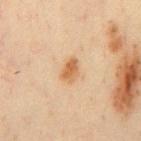No biopsy was performed on this lesion — it was imaged during a full skin examination and was not determined to be concerning.